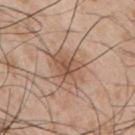Part of a total-body skin-imaging series; this lesion was reviewed on a skin check and was not flagged for biopsy. A male patient approximately 55 years of age. This image is a 15 mm lesion crop taken from a total-body photograph. The lesion is located on the arm.